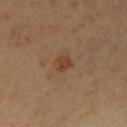No biopsy was performed on this lesion — it was imaged during a full skin examination and was not determined to be concerning.